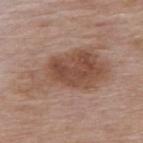Imaged during a routine full-body skin examination; the lesion was not biopsied and no histopathology is available.
A close-up tile cropped from a whole-body skin photograph, about 15 mm across.
From the back.
A male patient roughly 85 years of age or older.